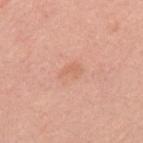notes: total-body-photography surveillance lesion; no biopsy
illumination: white-light illumination
imaging modality: ~15 mm tile from a whole-body skin photo
subject: female, approximately 55 years of age
diameter: ≈2.5 mm
location: the back